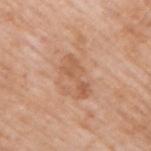  site: right upper arm
  lighting: white-light
  patient:
    sex: male
    age_approx: 60
  lesion_size:
    long_diameter_mm_approx: 5.0
  image:
    source: total-body photography crop
    field_of_view_mm: 15